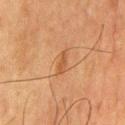Q: Was this lesion biopsied?
A: no biopsy performed (imaged during a skin exam)
Q: How was this image acquired?
A: total-body-photography crop, ~15 mm field of view
Q: What are the patient's age and sex?
A: male, aged 78 to 82
Q: Where on the body is the lesion?
A: the chest